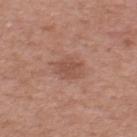The lesion was photographed on a routine skin check and not biopsied; there is no pathology result. Located on the upper back. Cropped from a whole-body photographic skin survey; the tile spans about 15 mm. This is a white-light tile. A male patient aged 53 to 57. The lesion-visualizer software estimated an eccentricity of roughly 0.7 and two-axis asymmetry of about 0.2. And it measured a lesion color around L≈51 a*≈22 b*≈28 in CIELAB and a normalized lesion–skin contrast near 5.5. The analysis additionally found a nevus-likeness score of about 0/100 and a lesion-detection confidence of about 100/100. About 3.5 mm across.